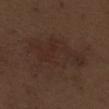biopsy status — total-body-photography surveillance lesion; no biopsy
image-analysis metrics — a footprint of about 26 mm², an outline eccentricity of about 0.8 (0 = round, 1 = elongated), and a symmetry-axis asymmetry near 0.35; a lesion-to-skin contrast of about 5 (normalized; higher = more distinct); a nevus-likeness score of about 10/100 and a lesion-detection confidence of about 100/100
body site — the right thigh
lesion diameter — about 8 mm
image — ~15 mm crop, total-body skin-cancer survey
tile lighting — white-light
subject — male, approximately 70 years of age